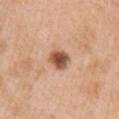follow-up: total-body-photography surveillance lesion; no biopsy | illumination: white-light | diameter: about 3 mm | imaging modality: total-body-photography crop, ~15 mm field of view | location: the right upper arm | patient: female, approximately 55 years of age.